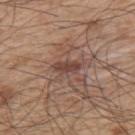Notes:
- follow-up — total-body-photography surveillance lesion; no biopsy
- tile lighting — white-light
- subject — male, about 50 years old
- site — the upper back
- acquisition — 15 mm crop, total-body photography